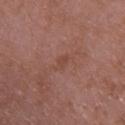This lesion was catalogued during total-body skin photography and was not selected for biopsy. Imaged with white-light lighting. Longest diameter approximately 2.5 mm. The lesion is on the upper back. The patient is a female aged around 70. Cropped from a total-body skin-imaging series; the visible field is about 15 mm. The lesion-visualizer software estimated a footprint of about 2.5 mm², an outline eccentricity of about 0.85 (0 = round, 1 = elongated), and two-axis asymmetry of about 0.3. It also reported a lesion color around L≈45 a*≈23 b*≈26 in CIELAB, about 5 CIELAB-L* units darker than the surrounding skin, and a normalized border contrast of about 4.5. The analysis additionally found a border-irregularity index near 3/10, a within-lesion color-variation index near 1/10, and peripheral color asymmetry of about 0.5. It also reported a nevus-likeness score of about 0/100 and lesion-presence confidence of about 100/100.